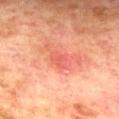{"biopsy_status": "not biopsied; imaged during a skin examination"}